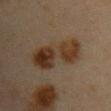<lesion>
  <patient>
    <sex>female</sex>
    <age_approx>45</age_approx>
  </patient>
  <site>arm</site>
  <image>
    <source>total-body photography crop</source>
    <field_of_view_mm>15</field_of_view_mm>
  </image>
</lesion>